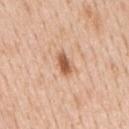<record>
  <biopsy_status>not biopsied; imaged during a skin examination</biopsy_status>
  <site>mid back</site>
  <image>
    <source>total-body photography crop</source>
    <field_of_view_mm>15</field_of_view_mm>
  </image>
  <patient>
    <sex>male</sex>
    <age_approx>65</age_approx>
  </patient>
</record>